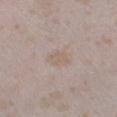Q: Was this lesion biopsied?
A: catalogued during a skin exam; not biopsied
Q: How was this image acquired?
A: ~15 mm crop, total-body skin-cancer survey
Q: Who is the patient?
A: female, aged 23 to 27
Q: How was the tile lit?
A: white-light illumination
Q: What is the anatomic site?
A: the left lower leg
Q: What is the lesion's diameter?
A: ~3 mm (longest diameter)